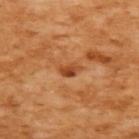Case summary:
- notes — total-body-photography surveillance lesion; no biopsy
- lesion diameter — ~3 mm (longest diameter)
- anatomic site — the upper back
- tile lighting — cross-polarized
- patient — female, aged 53 to 57
- acquisition — ~15 mm tile from a whole-body skin photo